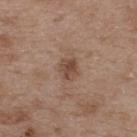Findings:
- workup — total-body-photography surveillance lesion; no biopsy
- patient — male, in their 50s
- lesion size — about 2.5 mm
- image — 15 mm crop, total-body photography
- lighting — white-light
- image-analysis metrics — a shape-asymmetry score of about 0.25 (0 = symmetric); a mean CIELAB color near L≈46 a*≈18 b*≈27
- location — the upper back